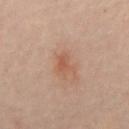The lesion was tiled from a total-body skin photograph and was not biopsied.
The tile uses cross-polarized illumination.
The patient is a male roughly 50 years of age.
The lesion-visualizer software estimated a lesion area of about 4 mm², a shape eccentricity near 0.7, and a symmetry-axis asymmetry near 0.3. It also reported a lesion–skin lightness drop of about 6. The analysis additionally found border irregularity of about 3.5 on a 0–10 scale, a color-variation rating of about 1.5/10, and a peripheral color-asymmetry measure near 0.5. The analysis additionally found a classifier nevus-likeness of about 5/100.
A region of skin cropped from a whole-body photographic capture, roughly 15 mm wide.
Longest diameter approximately 2.5 mm.
The lesion is on the mid back.